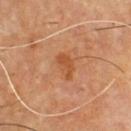notes: total-body-photography surveillance lesion; no biopsy | location: the chest | acquisition: 15 mm crop, total-body photography | subject: male, about 60 years old | lighting: cross-polarized illumination | diameter: ~3.5 mm (longest diameter) | TBP lesion metrics: a footprint of about 5 mm², a shape eccentricity near 0.85, and two-axis asymmetry of about 0.25; roughly 7 lightness units darker than nearby skin.